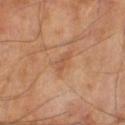Assessment: The lesion was photographed on a routine skin check and not biopsied; there is no pathology result. Acquisition and patient details: A roughly 15 mm field-of-view crop from a total-body skin photograph. An algorithmic analysis of the crop reported a footprint of about 2.5 mm² and a symmetry-axis asymmetry near 0.45. And it measured a lesion color around L≈52 a*≈22 b*≈34 in CIELAB and a lesion–skin lightness drop of about 7. The analysis additionally found border irregularity of about 4.5 on a 0–10 scale and radial color variation of about 0. Captured under cross-polarized illumination. About 3 mm across. A male patient, aged 63–67.